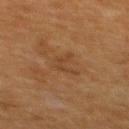Impression:
Imaged during a routine full-body skin examination; the lesion was not biopsied and no histopathology is available.
Context:
Captured under cross-polarized illumination. An algorithmic analysis of the crop reported a lesion area of about 3 mm² and a symmetry-axis asymmetry near 0.65. It also reported border irregularity of about 7.5 on a 0–10 scale, internal color variation of about 0 on a 0–10 scale, and peripheral color asymmetry of about 0. The analysis additionally found a nevus-likeness score of about 0/100 and a detector confidence of about 100 out of 100 that the crop contains a lesion. A 15 mm close-up tile from a total-body photography series done for melanoma screening. About 3 mm across. The lesion is located on the upper back. A female patient aged 53–57.